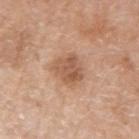Q: Was this lesion biopsied?
A: no biopsy performed (imaged during a skin exam)
Q: What kind of image is this?
A: ~15 mm crop, total-body skin-cancer survey
Q: Lesion location?
A: the left upper arm
Q: How was the tile lit?
A: white-light illumination
Q: Patient demographics?
A: male, aged 58–62
Q: What is the lesion's diameter?
A: ~4 mm (longest diameter)